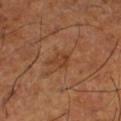Captured during whole-body skin photography for melanoma surveillance; the lesion was not biopsied.
The recorded lesion diameter is about 2.5 mm.
A 15 mm crop from a total-body photograph taken for skin-cancer surveillance.
A male patient roughly 65 years of age.
Automated image analysis of the tile measured a shape-asymmetry score of about 0.3 (0 = symmetric). It also reported an average lesion color of about L≈40 a*≈23 b*≈33 (CIELAB), about 7 CIELAB-L* units darker than the surrounding skin, and a normalized border contrast of about 6. It also reported a border-irregularity index near 3/10 and a color-variation rating of about 2/10.
The tile uses cross-polarized illumination.
On the left lower leg.